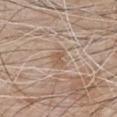Impression: Imaged during a routine full-body skin examination; the lesion was not biopsied and no histopathology is available. Acquisition and patient details: The total-body-photography lesion software estimated about 8 CIELAB-L* units darker than the surrounding skin and a lesion-to-skin contrast of about 6 (normalized; higher = more distinct). And it measured a border-irregularity rating of about 3.5/10, a color-variation rating of about 2/10, and a peripheral color-asymmetry measure near 0.5. And it measured lesion-presence confidence of about 100/100. On the chest. A 15 mm close-up tile from a total-body photography series done for melanoma screening. The tile uses white-light illumination. The lesion's longest dimension is about 2.5 mm. A male subject in their 80s.